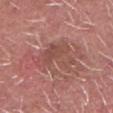Recorded during total-body skin imaging; not selected for excision or biopsy. A male subject, aged 58–62. On the head or neck. A 15 mm crop from a total-body photograph taken for skin-cancer surveillance. Automated image analysis of the tile measured an eccentricity of roughly 0.75. The software also gave a mean CIELAB color near L≈49 a*≈24 b*≈25, roughly 7 lightness units darker than nearby skin, and a lesion-to-skin contrast of about 5 (normalized; higher = more distinct). It also reported border irregularity of about 6 on a 0–10 scale and peripheral color asymmetry of about 1. The software also gave a classifier nevus-likeness of about 0/100. Captured under white-light illumination.